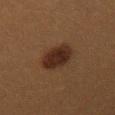Assessment: This lesion was catalogued during total-body skin photography and was not selected for biopsy. Acquisition and patient details: The subject is a female aged around 40. A 15 mm close-up tile from a total-body photography series done for melanoma screening. The lesion is on the right thigh. Longest diameter approximately 4.5 mm.